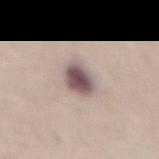An algorithmic analysis of the crop reported an eccentricity of roughly 0.55 and a shape-asymmetry score of about 0.15 (0 = symmetric). It also reported a border-irregularity rating of about 1.5/10, a color-variation rating of about 4.5/10, and a peripheral color-asymmetry measure near 1. On the abdomen. Cropped from a total-body skin-imaging series; the visible field is about 15 mm. The tile uses white-light illumination. A female subject aged 63 to 67.